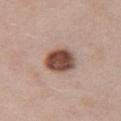Part of a total-body skin-imaging series; this lesion was reviewed on a skin check and was not flagged for biopsy. Cropped from a whole-body photographic skin survey; the tile spans about 15 mm. From the front of the torso. A female patient, roughly 50 years of age.